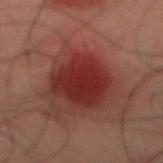| key | value |
|---|---|
| follow-up | imaged on a skin check; not biopsied |
| subject | male, approximately 50 years of age |
| lighting | cross-polarized illumination |
| location | the abdomen |
| size | about 6 mm |
| automated lesion analysis | an area of roughly 20 mm², an eccentricity of roughly 0.65, and a shape-asymmetry score of about 0.2 (0 = symmetric); a mean CIELAB color near L≈22 a*≈24 b*≈19, roughly 9 lightness units darker than nearby skin, and a normalized lesion–skin contrast near 10.5; a classifier nevus-likeness of about 85/100 and a lesion-detection confidence of about 100/100 |
| imaging modality | total-body-photography crop, ~15 mm field of view |